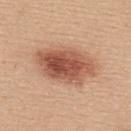The lesion was photographed on a routine skin check and not biopsied; there is no pathology result. A female subject, about 45 years old. On the upper back. A close-up tile cropped from a whole-body skin photograph, about 15 mm across.